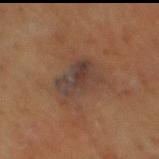Captured during whole-body skin photography for melanoma surveillance; the lesion was not biopsied. A 15 mm close-up extracted from a 3D total-body photography capture. A male subject, aged around 60. The lesion is located on the mid back.